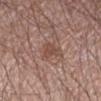<case>
  <patient>
    <sex>male</sex>
    <age_approx>55</age_approx>
  </patient>
  <site>arm</site>
  <image>
    <source>total-body photography crop</source>
    <field_of_view_mm>15</field_of_view_mm>
  </image>
</case>